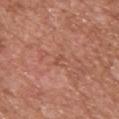<tbp_lesion>
  <biopsy_status>not biopsied; imaged during a skin examination</biopsy_status>
</tbp_lesion>